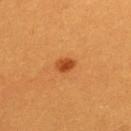{
  "biopsy_status": "not biopsied; imaged during a skin examination",
  "site": "back",
  "image": {
    "source": "total-body photography crop",
    "field_of_view_mm": 15
  },
  "patient": {
    "sex": "female",
    "age_approx": 30
  }
}